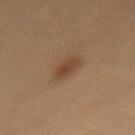Q: Was a biopsy performed?
A: catalogued during a skin exam; not biopsied
Q: What kind of image is this?
A: 15 mm crop, total-body photography
Q: How large is the lesion?
A: ≈2.5 mm
Q: What is the anatomic site?
A: the lower back
Q: What are the patient's age and sex?
A: female, approximately 50 years of age
Q: What did automated image analysis measure?
A: a nevus-likeness score of about 95/100 and lesion-presence confidence of about 100/100
Q: Illumination type?
A: cross-polarized